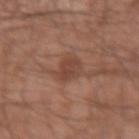The lesion was tiled from a total-body skin photograph and was not biopsied. Imaged with white-light lighting. The lesion's longest dimension is about 3 mm. A male patient, in their 30s. Cropped from a total-body skin-imaging series; the visible field is about 15 mm. Located on the right forearm.